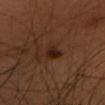Part of a total-body skin-imaging series; this lesion was reviewed on a skin check and was not flagged for biopsy. About 3 mm across. The total-body-photography lesion software estimated an area of roughly 5 mm², a shape eccentricity near 0.7, and two-axis asymmetry of about 0.25. It also reported a border-irregularity index near 2.5/10, a color-variation rating of about 4/10, and a peripheral color-asymmetry measure near 1. The software also gave a nevus-likeness score of about 70/100 and a detector confidence of about 100 out of 100 that the crop contains a lesion. A lesion tile, about 15 mm wide, cut from a 3D total-body photograph. From the head or neck. Imaged with cross-polarized lighting. A female subject, aged around 50.